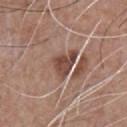This lesion was catalogued during total-body skin photography and was not selected for biopsy. A male patient in their mid-50s. This image is a 15 mm lesion crop taken from a total-body photograph. Imaged with white-light lighting. Longest diameter approximately 4 mm. The lesion is on the chest. The total-body-photography lesion software estimated internal color variation of about 4 on a 0–10 scale and a peripheral color-asymmetry measure near 1.5.